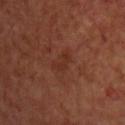{
  "biopsy_status": "not biopsied; imaged during a skin examination",
  "image": {
    "source": "total-body photography crop",
    "field_of_view_mm": 15
  },
  "patient": {
    "sex": "male",
    "age_approx": 65
  },
  "site": "front of the torso"
}